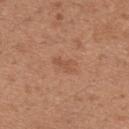biopsy status — catalogued during a skin exam; not biopsied | subject — female, approximately 30 years of age | tile lighting — white-light illumination | lesion size — ≈2.5 mm | body site — the left upper arm | imaging modality — total-body-photography crop, ~15 mm field of view | automated metrics — an eccentricity of roughly 0.85 and a symmetry-axis asymmetry near 0.5; an average lesion color of about L≈52 a*≈23 b*≈32 (CIELAB), a lesion–skin lightness drop of about 6, and a lesion-to-skin contrast of about 4.5 (normalized; higher = more distinct); a lesion-detection confidence of about 100/100.